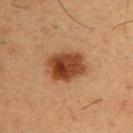Impression: Imaged during a routine full-body skin examination; the lesion was not biopsied and no histopathology is available. Acquisition and patient details: The lesion is located on the chest. A roughly 15 mm field-of-view crop from a total-body skin photograph. A male patient, roughly 55 years of age.